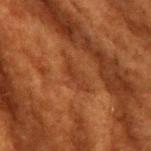  biopsy_status: not biopsied; imaged during a skin examination
  automated_metrics:
    area_mm2_approx: 5.0
    shape_asymmetry: 0.35
  lesion_size:
    long_diameter_mm_approx: 4.0
  site: front of the torso
  patient:
    sex: female
    age_approx: 80
  image:
    source: total-body photography crop
    field_of_view_mm: 15